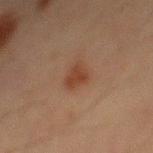Captured during whole-body skin photography for melanoma surveillance; the lesion was not biopsied.
A male subject, aged 68 to 72.
A 15 mm close-up extracted from a 3D total-body photography capture.
Located on the abdomen.